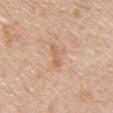A male subject, about 50 years old.
The total-body-photography lesion software estimated a lesion color around L≈63 a*≈19 b*≈33 in CIELAB, a lesion–skin lightness drop of about 7, and a normalized border contrast of about 5.5. The analysis additionally found border irregularity of about 3.5 on a 0–10 scale. The analysis additionally found a nevus-likeness score of about 0/100 and lesion-presence confidence of about 100/100.
The lesion is on the chest.
A region of skin cropped from a whole-body photographic capture, roughly 15 mm wide.
This is a white-light tile.
Longest diameter approximately 3.5 mm.